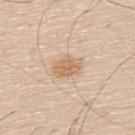The lesion was tiled from a total-body skin photograph and was not biopsied.
A male patient, approximately 60 years of age.
The lesion is on the upper back.
Automated image analysis of the tile measured an area of roughly 6.5 mm², an eccentricity of roughly 0.75, and a shape-asymmetry score of about 0.2 (0 = symmetric). And it measured an automated nevus-likeness rating near 65 out of 100 and lesion-presence confidence of about 100/100.
A 15 mm crop from a total-body photograph taken for skin-cancer surveillance.
Captured under white-light illumination.
The recorded lesion diameter is about 3.5 mm.